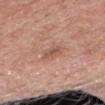Clinical impression: Recorded during total-body skin imaging; not selected for excision or biopsy. Context: The patient is a female aged 68 to 72. The recorded lesion diameter is about 2.5 mm. Imaged with white-light lighting. From the head or neck. A 15 mm close-up extracted from a 3D total-body photography capture. Automated image analysis of the tile measured border irregularity of about 3.5 on a 0–10 scale, a color-variation rating of about 1/10, and peripheral color asymmetry of about 0. And it measured a nevus-likeness score of about 0/100 and a detector confidence of about 100 out of 100 that the crop contains a lesion.